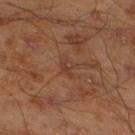Q: Is there a histopathology result?
A: catalogued during a skin exam; not biopsied
Q: What kind of image is this?
A: ~15 mm tile from a whole-body skin photo
Q: Patient demographics?
A: male, roughly 45 years of age
Q: Where on the body is the lesion?
A: the left lower leg
Q: Illumination type?
A: cross-polarized illumination
Q: Lesion size?
A: ~2.5 mm (longest diameter)